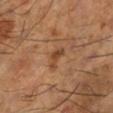Acquisition and patient details:
A male subject in their mid-60s. On the left lower leg. A roughly 15 mm field-of-view crop from a total-body skin photograph.